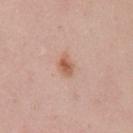Part of a total-body skin-imaging series; this lesion was reviewed on a skin check and was not flagged for biopsy.
The lesion is located on the left upper arm.
Cropped from a total-body skin-imaging series; the visible field is about 15 mm.
Approximately 2.5 mm at its widest.
A female patient, in their mid-20s.
The total-body-photography lesion software estimated a footprint of about 4 mm², a shape eccentricity near 0.7, and two-axis asymmetry of about 0.35. It also reported internal color variation of about 3 on a 0–10 scale and radial color variation of about 1. And it measured a classifier nevus-likeness of about 90/100 and a lesion-detection confidence of about 100/100.
Captured under white-light illumination.